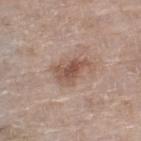follow-up — imaged on a skin check; not biopsied
TBP lesion metrics — a lesion area of about 9 mm² and a shape eccentricity near 0.8; a lesion color around L≈53 a*≈18 b*≈26 in CIELAB, a lesion–skin lightness drop of about 10, and a normalized border contrast of about 7.5; border irregularity of about 4.5 on a 0–10 scale, internal color variation of about 4 on a 0–10 scale, and radial color variation of about 1; a nevus-likeness score of about 65/100
lighting — white-light
site — the leg
lesion size — ≈4.5 mm
patient — female, approximately 75 years of age
acquisition — ~15 mm crop, total-body skin-cancer survey